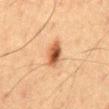<case>
<biopsy_status>not biopsied; imaged during a skin examination</biopsy_status>
<automated_metrics>
  <cielab_L>44</cielab_L>
  <cielab_a>21</cielab_a>
  <cielab_b>32</cielab_b>
  <vs_skin_darker_L>14.0</vs_skin_darker_L>
</automated_metrics>
<lighting>cross-polarized</lighting>
<site>mid back</site>
<patient>
  <sex>male</sex>
  <age_approx>60</age_approx>
</patient>
<lesion_size>
  <long_diameter_mm_approx>3.0</long_diameter_mm_approx>
</lesion_size>
<image>
  <source>total-body photography crop</source>
  <field_of_view_mm>15</field_of_view_mm>
</image>
</case>